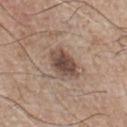| feature | finding |
|---|---|
| notes | no biopsy performed (imaged during a skin exam) |
| site | the chest |
| subject | male, aged around 75 |
| imaging modality | 15 mm crop, total-body photography |
| lesion diameter | about 4.5 mm |
| illumination | white-light |